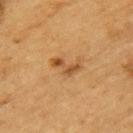<tbp_lesion>
<biopsy_status>not biopsied; imaged during a skin examination</biopsy_status>
</tbp_lesion>